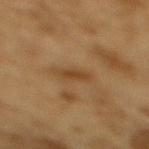No biopsy was performed on this lesion — it was imaged during a full skin examination and was not determined to be concerning. From the mid back. Measured at roughly 3 mm in maximum diameter. The tile uses cross-polarized illumination. The total-body-photography lesion software estimated a lesion area of about 3 mm², a shape eccentricity near 0.9, and a shape-asymmetry score of about 0.3 (0 = symmetric). Cropped from a total-body skin-imaging series; the visible field is about 15 mm. A male subject about 85 years old or older.